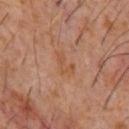Background:
Cropped from a whole-body photographic skin survey; the tile spans about 15 mm. A male subject approximately 60 years of age. The lesion is on the chest. Automated tile analysis of the lesion measured a classifier nevus-likeness of about 0/100 and a lesion-detection confidence of about 100/100. Captured under cross-polarized illumination. Longest diameter approximately 3 mm.